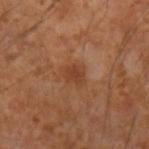| field | value |
|---|---|
| patient | male, in their mid-50s |
| site | the arm |
| lesion diameter | ≈2.5 mm |
| automated lesion analysis | a lesion area of about 4 mm², an outline eccentricity of about 0.6 (0 = round, 1 = elongated), and a symmetry-axis asymmetry near 0.25; about 8 CIELAB-L* units darker than the surrounding skin and a lesion-to-skin contrast of about 6.5 (normalized; higher = more distinct) |
| imaging modality | total-body-photography crop, ~15 mm field of view |
| tile lighting | cross-polarized illumination |